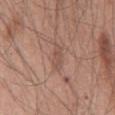Assessment:
Part of a total-body skin-imaging series; this lesion was reviewed on a skin check and was not flagged for biopsy.
Background:
This is a white-light tile. The lesion is on the chest. This image is a 15 mm lesion crop taken from a total-body photograph. The lesion's longest dimension is about 3.5 mm. A male subject, in their mid- to late 50s.